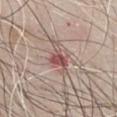Clinical impression:
This lesion was catalogued during total-body skin photography and was not selected for biopsy.
Context:
The lesion is located on the front of the torso. A roughly 15 mm field-of-view crop from a total-body skin photograph. A male patient aged 63 to 67. Measured at roughly 4 mm in maximum diameter. Captured under white-light illumination.